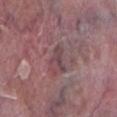workup: no biopsy performed (imaged during a skin exam)
site: the right lower leg
image-analysis metrics: an eccentricity of roughly 0.9 and a shape-asymmetry score of about 0.55 (0 = symmetric); a nevus-likeness score of about 0/100 and a detector confidence of about 50 out of 100 that the crop contains a lesion
tile lighting: white-light illumination
diameter: about 3.5 mm
acquisition: 15 mm crop, total-body photography
patient: male, approximately 40 years of age